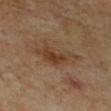Assessment: Imaged during a routine full-body skin examination; the lesion was not biopsied and no histopathology is available. Background: A lesion tile, about 15 mm wide, cut from a 3D total-body photograph. The lesion is located on the right lower leg. The subject is a male about 60 years old.